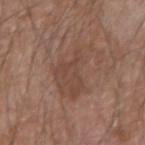Case summary:
• biopsy status — total-body-photography surveillance lesion; no biopsy
• lighting — white-light illumination
• TBP lesion metrics — an area of roughly 17 mm² and a symmetry-axis asymmetry near 0.5; a mean CIELAB color near L≈45 a*≈18 b*≈26, a lesion–skin lightness drop of about 6, and a normalized border contrast of about 5; a border-irregularity index near 6.5/10 and a peripheral color-asymmetry measure near 1
• patient — male, in their mid- to late 60s
• site — the arm
• image source — total-body-photography crop, ~15 mm field of view
• lesion size — ≈6 mm